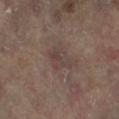Impression: Captured during whole-body skin photography for melanoma surveillance; the lesion was not biopsied.